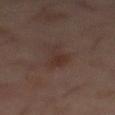Q: Was this lesion biopsied?
A: no biopsy performed (imaged during a skin exam)
Q: What are the patient's age and sex?
A: male, aged 58 to 62
Q: What lighting was used for the tile?
A: cross-polarized
Q: How was this image acquired?
A: ~15 mm tile from a whole-body skin photo
Q: What is the anatomic site?
A: the mid back
Q: How large is the lesion?
A: ~4.5 mm (longest diameter)